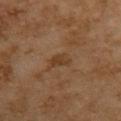tile lighting: cross-polarized illumination; site: the back; image: ~15 mm crop, total-body skin-cancer survey; patient: female, roughly 60 years of age; diameter: ≈3 mm.